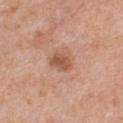Imaged during a routine full-body skin examination; the lesion was not biopsied and no histopathology is available. The tile uses white-light illumination. A female patient aged 43–47. Located on the chest. A region of skin cropped from a whole-body photographic capture, roughly 15 mm wide.